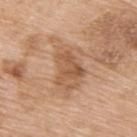Recorded during total-body skin imaging; not selected for excision or biopsy.
Captured under white-light illumination.
A close-up tile cropped from a whole-body skin photograph, about 15 mm across.
The total-body-photography lesion software estimated a lesion area of about 8 mm² and two-axis asymmetry of about 0.5. The software also gave a nevus-likeness score of about 0/100 and lesion-presence confidence of about 100/100.
A female subject, aged 73–77.
The lesion is on the right upper arm.
About 4 mm across.